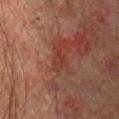  patient:
    sex: male
    age_approx: 75
  site: chest
  image:
    source: total-body photography crop
    field_of_view_mm: 15
  lesion_size:
    long_diameter_mm_approx: 3.5
  lighting: cross-polarized
  automated_metrics:
    cielab_L: 31
    cielab_a: 23
    cielab_b: 23
    vs_skin_darker_L: 6.0
    border_irregularity_0_10: 5.0
    color_variation_0_10: 1.5
    nevus_likeness_0_100: 0
    lesion_detection_confidence_0_100: 80